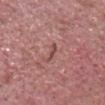Case summary:
– body site: the front of the torso
– patient: male, aged approximately 65
– image source: ~15 mm crop, total-body skin-cancer survey
– automated metrics: an area of roughly 2 mm², an outline eccentricity of about 0.95 (0 = round, 1 = elongated), and two-axis asymmetry of about 0.45; a lesion color around L≈48 a*≈23 b*≈23 in CIELAB; a classifier nevus-likeness of about 0/100 and a detector confidence of about 75 out of 100 that the crop contains a lesion